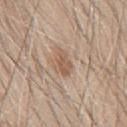notes=imaged on a skin check; not biopsied
patient=male, aged around 65
lighting=white-light illumination
site=the mid back
lesion diameter=≈3.5 mm
image source=15 mm crop, total-body photography